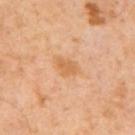| key | value |
|---|---|
| workup | no biopsy performed (imaged during a skin exam) |
| patient | male, approximately 65 years of age |
| automated lesion analysis | a footprint of about 4.5 mm² and a shape eccentricity near 0.75; a classifier nevus-likeness of about 0/100 |
| image | total-body-photography crop, ~15 mm field of view |
| illumination | cross-polarized illumination |
| lesion diameter | about 3 mm |